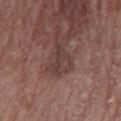Clinical impression: Captured during whole-body skin photography for melanoma surveillance; the lesion was not biopsied. Context: The lesion is located on the arm. A 15 mm close-up extracted from a 3D total-body photography capture. A female subject in their 70s. The total-body-photography lesion software estimated a footprint of about 7 mm², an outline eccentricity of about 0.85 (0 = round, 1 = elongated), and two-axis asymmetry of about 0.5. The software also gave a normalized lesion–skin contrast near 6. The analysis additionally found border irregularity of about 5.5 on a 0–10 scale and peripheral color asymmetry of about 1.5. It also reported an automated nevus-likeness rating near 0 out of 100 and a lesion-detection confidence of about 95/100. Approximately 4 mm at its widest. This is a white-light tile.